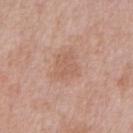  biopsy_status: not biopsied; imaged during a skin examination
  automated_metrics:
    cielab_L: 59
    cielab_a: 21
    cielab_b: 29
    vs_skin_darker_L: 6.0
    border_irregularity_0_10: 3.0
    color_variation_0_10: 2.0
    peripheral_color_asymmetry: 0.5
    nevus_likeness_0_100: 0
    lesion_detection_confidence_0_100: 100
  patient:
    sex: male
    age_approx: 50
  image:
    source: total-body photography crop
    field_of_view_mm: 15
  site: front of the torso
  lighting: white-light
  lesion_size:
    long_diameter_mm_approx: 3.5